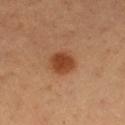Q: Was this lesion biopsied?
A: total-body-photography surveillance lesion; no biopsy
Q: How was this image acquired?
A: total-body-photography crop, ~15 mm field of view
Q: Patient demographics?
A: male, aged approximately 50
Q: What lighting was used for the tile?
A: cross-polarized illumination
Q: Lesion location?
A: the left thigh